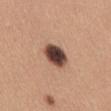Q: Is there a histopathology result?
A: catalogued during a skin exam; not biopsied
Q: Lesion location?
A: the abdomen
Q: What lighting was used for the tile?
A: white-light illumination
Q: What are the patient's age and sex?
A: female, aged approximately 30
Q: What is the imaging modality?
A: ~15 mm tile from a whole-body skin photo
Q: What is the lesion's diameter?
A: ≈3.5 mm